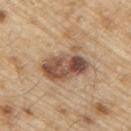{
  "biopsy_status": "not biopsied; imaged during a skin examination",
  "automated_metrics": {
    "cielab_L": 52,
    "cielab_a": 18,
    "cielab_b": 29,
    "border_irregularity_0_10": 3.5,
    "color_variation_0_10": 8.5,
    "nevus_likeness_0_100": 75,
    "lesion_detection_confidence_0_100": 100
  },
  "lighting": "white-light",
  "site": "right upper arm",
  "image": {
    "source": "total-body photography crop",
    "field_of_view_mm": 15
  },
  "patient": {
    "sex": "male",
    "age_approx": 70
  },
  "lesion_size": {
    "long_diameter_mm_approx": 5.5
  }
}